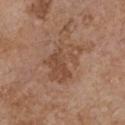• notes · total-body-photography surveillance lesion; no biopsy